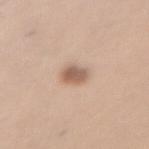Q: Is there a histopathology result?
A: catalogued during a skin exam; not biopsied
Q: What did automated image analysis measure?
A: two-axis asymmetry of about 0.25; a mean CIELAB color near L≈59 a*≈18 b*≈28, roughly 12 lightness units darker than nearby skin, and a normalized border contrast of about 8
Q: Lesion size?
A: ≈3 mm
Q: Patient demographics?
A: female, aged approximately 45
Q: Illumination type?
A: white-light
Q: Lesion location?
A: the abdomen
Q: What kind of image is this?
A: ~15 mm crop, total-body skin-cancer survey